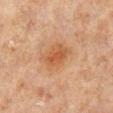Captured during whole-body skin photography for melanoma surveillance; the lesion was not biopsied.
A region of skin cropped from a whole-body photographic capture, roughly 15 mm wide.
The lesion is located on the right lower leg.
Captured under cross-polarized illumination.
A male patient approximately 70 years of age.
An algorithmic analysis of the crop reported a footprint of about 11 mm², a shape eccentricity near 0.55, and a shape-asymmetry score of about 0.2 (0 = symmetric). The analysis additionally found internal color variation of about 3.5 on a 0–10 scale and a peripheral color-asymmetry measure near 1.